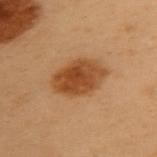Recorded during total-body skin imaging; not selected for excision or biopsy.
A close-up tile cropped from a whole-body skin photograph, about 15 mm across.
On the back.
A female patient, aged approximately 60.
The tile uses cross-polarized illumination.
Approximately 6 mm at its widest.